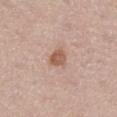Assessment: This lesion was catalogued during total-body skin photography and was not selected for biopsy. Background: A region of skin cropped from a whole-body photographic capture, roughly 15 mm wide. On the right thigh. A male subject about 75 years old.